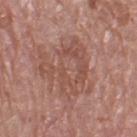No biopsy was performed on this lesion — it was imaged during a full skin examination and was not determined to be concerning.
The lesion-visualizer software estimated a border-irregularity rating of about 8.5/10 and a color-variation rating of about 5/10. It also reported an automated nevus-likeness rating near 0 out of 100.
About 7 mm across.
The lesion is located on the left thigh.
A close-up tile cropped from a whole-body skin photograph, about 15 mm across.
Imaged with white-light lighting.
The patient is a female aged around 55.